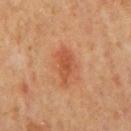Clinical impression:
This lesion was catalogued during total-body skin photography and was not selected for biopsy.
Background:
About 4 mm across. From the mid back. A male patient about 65 years old. Captured under cross-polarized illumination. A region of skin cropped from a whole-body photographic capture, roughly 15 mm wide.